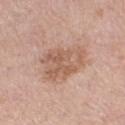follow-up: no biopsy performed (imaged during a skin exam) | tile lighting: white-light illumination | image-analysis metrics: a lesion area of about 17 mm², an outline eccentricity of about 0.65 (0 = round, 1 = elongated), and two-axis asymmetry of about 0.3; a lesion color around L≈59 a*≈20 b*≈29 in CIELAB, a lesion–skin lightness drop of about 9, and a normalized lesion–skin contrast near 6.5 | patient: female, aged approximately 40 | acquisition: total-body-photography crop, ~15 mm field of view | location: the right thigh.